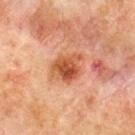No biopsy was performed on this lesion — it was imaged during a full skin examination and was not determined to be concerning. A 15 mm close-up extracted from a 3D total-body photography capture. A male patient aged around 70. Automated image analysis of the tile measured about 11 CIELAB-L* units darker than the surrounding skin. It also reported border irregularity of about 2.5 on a 0–10 scale and a within-lesion color-variation index near 6/10. The software also gave a lesion-detection confidence of about 100/100. Located on the upper back.